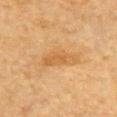Impression:
The lesion was tiled from a total-body skin photograph and was not biopsied.
Background:
From the chest. A 15 mm close-up tile from a total-body photography series done for melanoma screening. A male patient aged 83 or older. Imaged with cross-polarized lighting. Automated image analysis of the tile measured an outline eccentricity of about 0.9 (0 = round, 1 = elongated) and a shape-asymmetry score of about 0.4 (0 = symmetric). The software also gave border irregularity of about 4.5 on a 0–10 scale, a color-variation rating of about 3/10, and peripheral color asymmetry of about 1. The software also gave an automated nevus-likeness rating near 0 out of 100 and lesion-presence confidence of about 100/100. The recorded lesion diameter is about 5.5 mm.